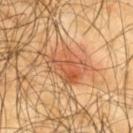Q: Was this lesion biopsied?
A: total-body-photography surveillance lesion; no biopsy
Q: What did automated image analysis measure?
A: a footprint of about 13 mm², a shape eccentricity near 0.75, and a symmetry-axis asymmetry near 0.35; a mean CIELAB color near L≈54 a*≈25 b*≈37 and a normalized border contrast of about 6.5
Q: Where on the body is the lesion?
A: the upper back
Q: Who is the patient?
A: male, roughly 65 years of age
Q: How large is the lesion?
A: ~5.5 mm (longest diameter)
Q: What kind of image is this?
A: 15 mm crop, total-body photography
Q: How was the tile lit?
A: cross-polarized